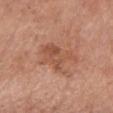notes: no biopsy performed (imaged during a skin exam); subject: female, aged 73 to 77; TBP lesion metrics: a footprint of about 12 mm², an outline eccentricity of about 0.85 (0 = round, 1 = elongated), and two-axis asymmetry of about 0.4; tile lighting: white-light illumination; image source: ~15 mm tile from a whole-body skin photo; body site: the mid back; lesion size: ~6 mm (longest diameter).